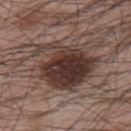notes: no biopsy performed (imaged during a skin exam)
lighting: white-light illumination
patient: male, aged around 65
anatomic site: the left upper arm
image: ~15 mm tile from a whole-body skin photo
automated lesion analysis: a footprint of about 29 mm², an outline eccentricity of about 0.6 (0 = round, 1 = elongated), and two-axis asymmetry of about 0.3